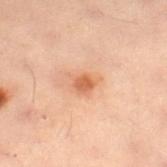Notes:
– follow-up · catalogued during a skin exam; not biopsied
– subject · female, aged 28 to 32
– image · total-body-photography crop, ~15 mm field of view
– tile lighting · cross-polarized illumination
– body site · the left leg